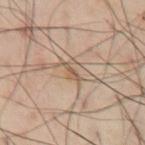<record>
<biopsy_status>not biopsied; imaged during a skin examination</biopsy_status>
<image>
  <source>total-body photography crop</source>
  <field_of_view_mm>15</field_of_view_mm>
</image>
<site>abdomen</site>
<lesion_size>
  <long_diameter_mm_approx>2.5</long_diameter_mm_approx>
</lesion_size>
<automated_metrics>
  <border_irregularity_0_10>4.0</border_irregularity_0_10>
  <peripheral_color_asymmetry>0.0</peripheral_color_asymmetry>
  <nevus_likeness_0_100>0</nevus_likeness_0_100>
  <lesion_detection_confidence_0_100>50</lesion_detection_confidence_0_100>
</automated_metrics>
<lighting>cross-polarized</lighting>
<patient>
  <sex>male</sex>
  <age_approx>55</age_approx>
</patient>
</record>